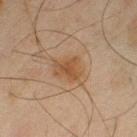Imaged during a routine full-body skin examination; the lesion was not biopsied and no histopathology is available. The lesion is located on the leg. This is a cross-polarized tile. Automated tile analysis of the lesion measured a mean CIELAB color near L≈41 a*≈16 b*≈29 and a lesion–skin lightness drop of about 7. The software also gave border irregularity of about 2.5 on a 0–10 scale and peripheral color asymmetry of about 1. The analysis additionally found a nevus-likeness score of about 50/100 and lesion-presence confidence of about 100/100. A male subject, aged 43–47. A 15 mm crop from a total-body photograph taken for skin-cancer surveillance.